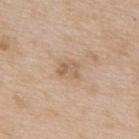biopsy status=no biopsy performed (imaged during a skin exam); subject=male, aged approximately 65; image source=~15 mm crop, total-body skin-cancer survey; anatomic site=the upper back; diameter=about 2.5 mm; tile lighting=white-light.